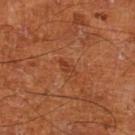Imaged during a routine full-body skin examination; the lesion was not biopsied and no histopathology is available. This is a cross-polarized tile. Longest diameter approximately 2.5 mm. Automated image analysis of the tile measured a footprint of about 2.5 mm² and an outline eccentricity of about 0.9 (0 = round, 1 = elongated). And it measured an average lesion color of about L≈38 a*≈27 b*≈34 (CIELAB) and a normalized border contrast of about 5.5. It also reported an automated nevus-likeness rating near 0 out of 100. On the left lower leg. A male patient aged approximately 65. A roughly 15 mm field-of-view crop from a total-body skin photograph.